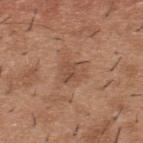Impression:
Recorded during total-body skin imaging; not selected for excision or biopsy.
Context:
Located on the upper back. Cropped from a whole-body photographic skin survey; the tile spans about 15 mm. A male subject approximately 40 years of age.